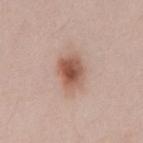Q: Is there a histopathology result?
A: imaged on a skin check; not biopsied
Q: What kind of image is this?
A: 15 mm crop, total-body photography
Q: Who is the patient?
A: male, aged 33 to 37
Q: Lesion size?
A: ~4 mm (longest diameter)
Q: Lesion location?
A: the mid back
Q: Automated lesion metrics?
A: a lesion area of about 9 mm² and an outline eccentricity of about 0.7 (0 = round, 1 = elongated); a mean CIELAB color near L≈55 a*≈21 b*≈28 and a lesion–skin lightness drop of about 13; border irregularity of about 2 on a 0–10 scale and peripheral color asymmetry of about 1.5